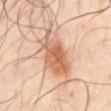image:
  source: total-body photography crop
  field_of_view_mm: 15
site: chest
lesion_size:
  long_diameter_mm_approx: 6.5
lighting: cross-polarized
patient:
  sex: male
  age_approx: 65
automated_metrics:
  vs_skin_darker_L: 12.0
  vs_skin_contrast_norm: 8.0
  border_irregularity_0_10: 4.0
  color_variation_0_10: 6.0
  peripheral_color_asymmetry: 2.0